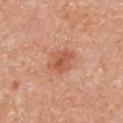follow-up: total-body-photography surveillance lesion; no biopsy
image: 15 mm crop, total-body photography
patient: female, in their mid-70s
body site: the right upper arm